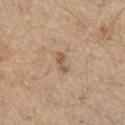site=the front of the torso
size=≈2.5 mm
TBP lesion metrics=a lesion–skin lightness drop of about 10 and a normalized lesion–skin contrast near 7; a border-irregularity rating of about 3.5/10 and a within-lesion color-variation index near 1.5/10; an automated nevus-likeness rating near 5 out of 100 and a detector confidence of about 100 out of 100 that the crop contains a lesion
patient=male, aged 68 to 72
imaging modality=~15 mm tile from a whole-body skin photo